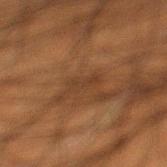<tbp_lesion>
  <biopsy_status>not biopsied; imaged during a skin examination</biopsy_status>
  <image>
    <source>total-body photography crop</source>
    <field_of_view_mm>15</field_of_view_mm>
  </image>
  <site>left lower leg</site>
  <patient>
    <sex>male</sex>
    <age_approx>50</age_approx>
  </patient>
</tbp_lesion>